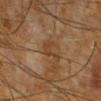Case summary:
- follow-up: imaged on a skin check; not biopsied
- tile lighting: cross-polarized
- patient: male, roughly 65 years of age
- acquisition: total-body-photography crop, ~15 mm field of view
- location: the right lower leg
- lesion diameter: ≈3 mm